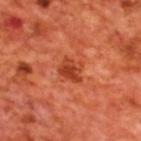The lesion was photographed on a routine skin check and not biopsied; there is no pathology result.
Imaged with cross-polarized lighting.
A lesion tile, about 15 mm wide, cut from a 3D total-body photograph.
A male patient approximately 70 years of age.
Located on the upper back.
Approximately 3 mm at its widest.